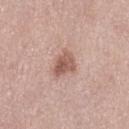Clinical summary: The tile uses white-light illumination. A female subject aged 38 to 42. Approximately 3.5 mm at its widest. A roughly 15 mm field-of-view crop from a total-body skin photograph. Located on the leg.